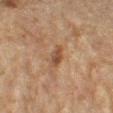Case summary:
• workup: catalogued during a skin exam; not biopsied
• image: total-body-photography crop, ~15 mm field of view
• location: the left forearm
• subject: female, aged 78 to 82
• diameter: about 3.5 mm